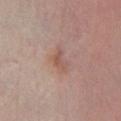A male subject, about 65 years old. About 3 mm across. Located on the leg. A lesion tile, about 15 mm wide, cut from a 3D total-body photograph. Automated tile analysis of the lesion measured a lesion–skin lightness drop of about 6 and a normalized border contrast of about 5.5.